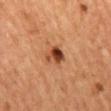| feature | finding |
|---|---|
| workup | no biopsy performed (imaged during a skin exam) |
| diameter | ~3 mm (longest diameter) |
| image-analysis metrics | a footprint of about 5 mm², an eccentricity of roughly 0.65, and a shape-asymmetry score of about 0.3 (0 = symmetric); a detector confidence of about 100 out of 100 that the crop contains a lesion |
| subject | female, about 60 years old |
| tile lighting | cross-polarized illumination |
| acquisition | ~15 mm tile from a whole-body skin photo |
| body site | the mid back |